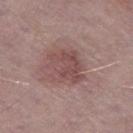The lesion was tiled from a total-body skin photograph and was not biopsied. The tile uses white-light illumination. Cropped from a whole-body photographic skin survey; the tile spans about 15 mm. On the left thigh. A male patient aged 73–77. Measured at roughly 4.5 mm in maximum diameter. An algorithmic analysis of the crop reported a lesion color around L≈48 a*≈21 b*≈19 in CIELAB, roughly 8 lightness units darker than nearby skin, and a normalized border contrast of about 6. And it measured a border-irregularity index near 4/10, internal color variation of about 3.5 on a 0–10 scale, and radial color variation of about 1.